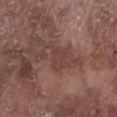The lesion is located on the left lower leg.
The subject is a male approximately 75 years of age.
This image is a 15 mm lesion crop taken from a total-body photograph.